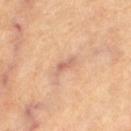This lesion was catalogued during total-body skin photography and was not selected for biopsy.
On the left thigh.
The patient is a female about 65 years old.
A 15 mm crop from a total-body photograph taken for skin-cancer surveillance.